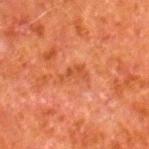- biopsy status — total-body-photography surveillance lesion; no biopsy
- automated metrics — a footprint of about 4 mm², an outline eccentricity of about 0.85 (0 = round, 1 = elongated), and two-axis asymmetry of about 0.5; border irregularity of about 5.5 on a 0–10 scale, internal color variation of about 1 on a 0–10 scale, and a peripheral color-asymmetry measure near 0
- lighting — cross-polarized illumination
- subject — male, aged around 80
- lesion diameter — ≈3 mm
- body site — the leg
- acquisition — 15 mm crop, total-body photography